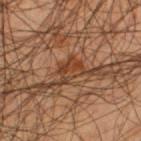Case summary:
• biopsy status: no biopsy performed (imaged during a skin exam)
• imaging modality: ~15 mm crop, total-body skin-cancer survey
• diameter: about 3.5 mm
• patient: male, aged 53–57
• tile lighting: cross-polarized
• body site: the left thigh